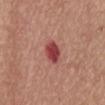{"biopsy_status": "not biopsied; imaged during a skin examination", "image": {"source": "total-body photography crop", "field_of_view_mm": 15}, "site": "abdomen", "patient": {"sex": "female", "age_approx": 75}}